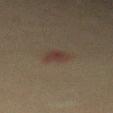Clinical impression:
Imaged during a routine full-body skin examination; the lesion was not biopsied and no histopathology is available.